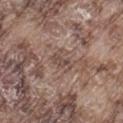Clinical impression: Imaged during a routine full-body skin examination; the lesion was not biopsied and no histopathology is available. Image and clinical context: This is a white-light tile. A male patient in their mid- to late 70s. From the left thigh. A region of skin cropped from a whole-body photographic capture, roughly 15 mm wide. The lesion-visualizer software estimated a lesion color around L≈47 a*≈16 b*≈22 in CIELAB, a lesion–skin lightness drop of about 8, and a lesion-to-skin contrast of about 6.5 (normalized; higher = more distinct).